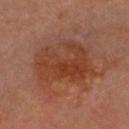Clinical impression: Imaged during a routine full-body skin examination; the lesion was not biopsied and no histopathology is available. Image and clinical context: A region of skin cropped from a whole-body photographic capture, roughly 15 mm wide. The subject is a male aged around 60. The lesion is on the head or neck.